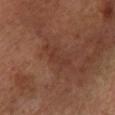Q: Is there a histopathology result?
A: total-body-photography surveillance lesion; no biopsy
Q: How was this image acquired?
A: ~15 mm crop, total-body skin-cancer survey
Q: What are the patient's age and sex?
A: male, about 65 years old
Q: What is the anatomic site?
A: the left lower leg